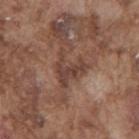Findings:
– follow-up: total-body-photography surveillance lesion; no biopsy
– lighting: white-light illumination
– lesion diameter: ~4 mm (longest diameter)
– image source: 15 mm crop, total-body photography
– anatomic site: the mid back
– subject: male, about 75 years old